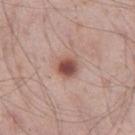Findings:
– subject: male, in their mid-50s
– acquisition: ~15 mm crop, total-body skin-cancer survey
– body site: the right thigh